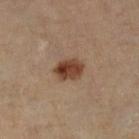Recorded during total-body skin imaging; not selected for excision or biopsy. A lesion tile, about 15 mm wide, cut from a 3D total-body photograph. On the left lower leg. Captured under cross-polarized illumination. About 3.5 mm across. The subject is a female roughly 60 years of age.